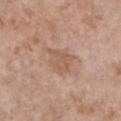Clinical impression: Captured during whole-body skin photography for melanoma surveillance; the lesion was not biopsied. Background: On the chest. A 15 mm crop from a total-body photograph taken for skin-cancer surveillance. An algorithmic analysis of the crop reported a footprint of about 8 mm², an eccentricity of roughly 0.65, and a symmetry-axis asymmetry near 0.3. The analysis additionally found a lesion color around L≈57 a*≈19 b*≈29 in CIELAB, a lesion–skin lightness drop of about 7, and a normalized border contrast of about 5.5. About 4 mm across. A female patient aged 83 or older.